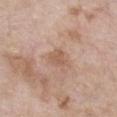Impression: This lesion was catalogued during total-body skin photography and was not selected for biopsy. Image and clinical context: Approximately 2.5 mm at its widest. The patient is a female aged around 75. A 15 mm close-up extracted from a 3D total-body photography capture. This is a white-light tile. Located on the chest.